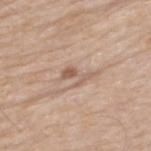Imaged during a routine full-body skin examination; the lesion was not biopsied and no histopathology is available. Located on the upper back. The tile uses white-light illumination. A male patient aged around 65. The recorded lesion diameter is about 3.5 mm. An algorithmic analysis of the crop reported a lesion area of about 4 mm², an outline eccentricity of about 0.85 (0 = round, 1 = elongated), and two-axis asymmetry of about 0.55. The analysis additionally found a border-irregularity index near 8.5/10 and peripheral color asymmetry of about 0. The analysis additionally found an automated nevus-likeness rating near 0 out of 100 and lesion-presence confidence of about 95/100. A 15 mm crop from a total-body photograph taken for skin-cancer surveillance.